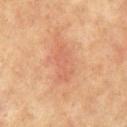Assessment: Captured during whole-body skin photography for melanoma surveillance; the lesion was not biopsied. Background: A male subject aged 63–67. Measured at roughly 5 mm in maximum diameter. Located on the chest. This image is a 15 mm lesion crop taken from a total-body photograph. This is a cross-polarized tile.